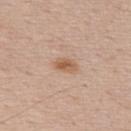| field | value |
|---|---|
| notes | imaged on a skin check; not biopsied |
| lesion size | ~2.5 mm (longest diameter) |
| subject | male, aged 53–57 |
| imaging modality | total-body-photography crop, ~15 mm field of view |
| automated lesion analysis | a footprint of about 4 mm², a shape eccentricity near 0.75, and a symmetry-axis asymmetry near 0.2; a within-lesion color-variation index near 3/10; a classifier nevus-likeness of about 80/100 |
| tile lighting | white-light illumination |